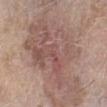biopsy status: imaged on a skin check; not biopsied | lesion diameter: ≈11 mm | patient: female, in their mid- to late 70s | site: the leg | lighting: white-light | imaging modality: 15 mm crop, total-body photography.